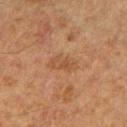biopsy status = total-body-photography surveillance lesion; no biopsy
site = the right forearm
subject = male, aged 63 to 67
acquisition = ~15 mm crop, total-body skin-cancer survey